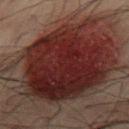No biopsy was performed on this lesion — it was imaged during a full skin examination and was not determined to be concerning.
Located on the lower back.
This image is a 15 mm lesion crop taken from a total-body photograph.
A male patient aged 48–52.